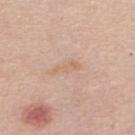automated lesion analysis = an area of roughly 3 mm² and a shape eccentricity near 0.95; an average lesion color of about L≈65 a*≈18 b*≈32 (CIELAB), a lesion–skin lightness drop of about 6, and a normalized border contrast of about 5.5; a border-irregularity rating of about 6/10, a color-variation rating of about 0/10, and peripheral color asymmetry of about 0; a classifier nevus-likeness of about 0/100 and a lesion-detection confidence of about 100/100
lesion size = ≈3.5 mm
tile lighting = white-light illumination
subject = female, aged around 55
body site = the upper back
image = ~15 mm crop, total-body skin-cancer survey